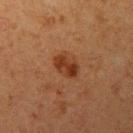Case summary:
• workup · imaged on a skin check; not biopsied
• site · the left upper arm
• acquisition · total-body-photography crop, ~15 mm field of view
• subject · female, approximately 40 years of age